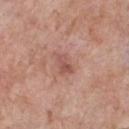follow-up=catalogued during a skin exam; not biopsied | diameter=≈3 mm | subject=male, aged approximately 60 | lighting=white-light illumination | site=the chest | image=15 mm crop, total-body photography.